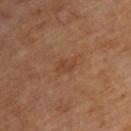follow-up = imaged on a skin check; not biopsied
image source = ~15 mm tile from a whole-body skin photo
site = the upper back
patient = male, aged 58 to 62
size = about 3 mm
automated lesion analysis = an average lesion color of about L≈41 a*≈21 b*≈31 (CIELAB) and roughly 5 lightness units darker than nearby skin